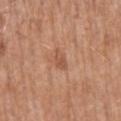Impression: Imaged during a routine full-body skin examination; the lesion was not biopsied and no histopathology is available. Context: The total-body-photography lesion software estimated an average lesion color of about L≈54 a*≈23 b*≈32 (CIELAB), about 8 CIELAB-L* units darker than the surrounding skin, and a normalized lesion–skin contrast near 6. The analysis additionally found a border-irregularity index near 3.5/10, internal color variation of about 1 on a 0–10 scale, and radial color variation of about 0.5. The software also gave a classifier nevus-likeness of about 0/100 and lesion-presence confidence of about 100/100. Located on the mid back. The patient is a male aged 68–72. This image is a 15 mm lesion crop taken from a total-body photograph.